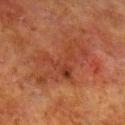biopsy status: total-body-photography surveillance lesion; no biopsy
anatomic site: the right lower leg
image source: 15 mm crop, total-body photography
illumination: cross-polarized
patient: male, in their 80s